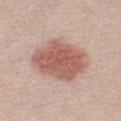Q: Was this lesion biopsied?
A: imaged on a skin check; not biopsied
Q: Automated lesion metrics?
A: an area of roughly 26 mm², an eccentricity of roughly 0.65, and a symmetry-axis asymmetry near 0.15; roughly 13 lightness units darker than nearby skin and a normalized lesion–skin contrast near 8.5; a border-irregularity index near 1.5/10, a color-variation rating of about 4/10, and peripheral color asymmetry of about 1
Q: What is the lesion's diameter?
A: ~6.5 mm (longest diameter)
Q: What is the imaging modality?
A: total-body-photography crop, ~15 mm field of view
Q: Patient demographics?
A: male, approximately 30 years of age
Q: How was the tile lit?
A: white-light